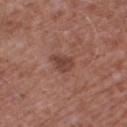<tbp_lesion>
<biopsy_status>not biopsied; imaged during a skin examination</biopsy_status>
<automated_metrics>
  <area_mm2_approx>4.0</area_mm2_approx>
  <eccentricity>0.75</eccentricity>
  <shape_asymmetry>0.35</shape_asymmetry>
  <nevus_likeness_0_100>10</nevus_likeness_0_100>
  <lesion_detection_confidence_0_100>100</lesion_detection_confidence_0_100>
</automated_metrics>
<lesion_size>
  <long_diameter_mm_approx>3.0</long_diameter_mm_approx>
</lesion_size>
<site>left thigh</site>
<image>
  <source>total-body photography crop</source>
  <field_of_view_mm>15</field_of_view_mm>
</image>
<lighting>white-light</lighting>
<patient>
  <sex>male</sex>
  <age_approx>60</age_approx>
</patient>
</tbp_lesion>